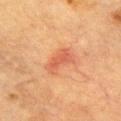<case>
<biopsy_status>not biopsied; imaged during a skin examination</biopsy_status>
</case>